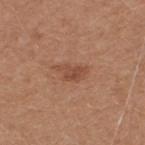Longest diameter approximately 3.5 mm. The lesion is on the upper back. This image is a 15 mm lesion crop taken from a total-body photograph. A male patient in their mid- to late 60s.